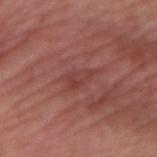{"site": "right forearm", "patient": {"sex": "female", "age_approx": 50}, "image": {"source": "total-body photography crop", "field_of_view_mm": 15}}